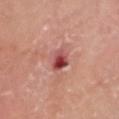Notes:
* biopsy status · no biopsy performed (imaged during a skin exam)
* lighting · white-light illumination
* TBP lesion metrics · a lesion–skin lightness drop of about 14 and a lesion-to-skin contrast of about 9.5 (normalized; higher = more distinct); an automated nevus-likeness rating near 0 out of 100 and lesion-presence confidence of about 100/100
* image · total-body-photography crop, ~15 mm field of view
* diameter · ≈3 mm
* subject · male, roughly 65 years of age
* anatomic site · the head or neck